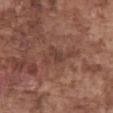Clinical impression: This lesion was catalogued during total-body skin photography and was not selected for biopsy. Background: From the abdomen. This is a white-light tile. This image is a 15 mm lesion crop taken from a total-body photograph. A male subject, in their mid- to late 70s.